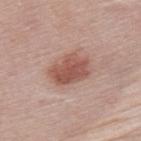Case summary:
– workup · total-body-photography surveillance lesion; no biopsy
– image source · ~15 mm tile from a whole-body skin photo
– patient · female, roughly 50 years of age
– body site · the upper back